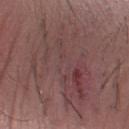Recorded during total-body skin imaging; not selected for excision or biopsy. The recorded lesion diameter is about 11 mm. On the right forearm. Automated tile analysis of the lesion measured an area of roughly 47 mm² and an outline eccentricity of about 0.75 (0 = round, 1 = elongated). The analysis additionally found border irregularity of about 8 on a 0–10 scale, a color-variation rating of about 6.5/10, and radial color variation of about 2. A male subject, roughly 45 years of age. A close-up tile cropped from a whole-body skin photograph, about 15 mm across. The tile uses white-light illumination.